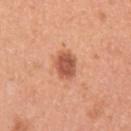| field | value |
|---|---|
| biopsy status | no biopsy performed (imaged during a skin exam) |
| location | the left upper arm |
| automated metrics | a lesion area of about 7 mm², a shape eccentricity near 0.45, and a symmetry-axis asymmetry near 0.2; a classifier nevus-likeness of about 90/100 and lesion-presence confidence of about 100/100 |
| lighting | white-light illumination |
| subject | female, aged around 35 |
| lesion diameter | about 3 mm |
| image | total-body-photography crop, ~15 mm field of view |